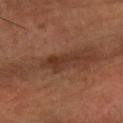No biopsy was performed on this lesion — it was imaged during a full skin examination and was not determined to be concerning.